The lesion is located on the upper back; a roughly 15 mm field-of-view crop from a total-body skin photograph; a male subject, in their mid-30s.
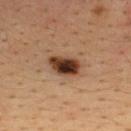<lesion>
  <lesion_size>
    <long_diameter_mm_approx>4.0</long_diameter_mm_approx>
  </lesion_size>
  <lighting>cross-polarized</lighting>
  <diagnosis>
    <histopathology>atypical melanocytic neoplasm</histopathology>
    <malignancy>indeterminate</malignancy>
    <taxonomic_path>Indeterminate, Indeterminate melanocytic proliferations, Atypical melanocytic neoplasm</taxonomic_path>
  </diagnosis>
</lesion>